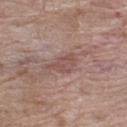No biopsy was performed on this lesion — it was imaged during a full skin examination and was not determined to be concerning.
The patient is a female aged around 70.
Measured at roughly 3.5 mm in maximum diameter.
Cropped from a total-body skin-imaging series; the visible field is about 15 mm.
From the left thigh.